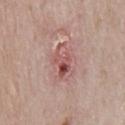Captured during whole-body skin photography for melanoma surveillance; the lesion was not biopsied. A male subject about 80 years old. A 15 mm close-up tile from a total-body photography series done for melanoma screening. On the chest. The recorded lesion diameter is about 4.5 mm.